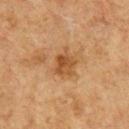The lesion was tiled from a total-body skin photograph and was not biopsied.
This is a cross-polarized tile.
Located on the chest.
An algorithmic analysis of the crop reported an average lesion color of about L≈40 a*≈19 b*≈33 (CIELAB), about 9 CIELAB-L* units darker than the surrounding skin, and a normalized lesion–skin contrast near 7.5. The software also gave an automated nevus-likeness rating near 10 out of 100 and lesion-presence confidence of about 100/100.
Measured at roughly 3.5 mm in maximum diameter.
A male patient, aged 73–77.
A 15 mm close-up extracted from a 3D total-body photography capture.